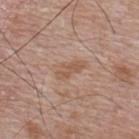Assessment:
This lesion was catalogued during total-body skin photography and was not selected for biopsy.
Context:
The patient is a male about 65 years old. The tile uses white-light illumination. Cropped from a whole-body photographic skin survey; the tile spans about 15 mm. Located on the back. The recorded lesion diameter is about 4 mm.